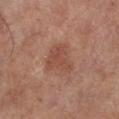The lesion was tiled from a total-body skin photograph and was not biopsied. A lesion tile, about 15 mm wide, cut from a 3D total-body photograph. The subject is a male aged 68 to 72. Approximately 4 mm at its widest. Captured under white-light illumination. From the left lower leg.